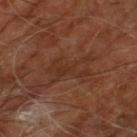follow-up: no biopsy performed (imaged during a skin exam); patient: male, approximately 60 years of age; image: ~15 mm tile from a whole-body skin photo; anatomic site: the right leg.